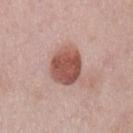Q: Was this lesion biopsied?
A: catalogued during a skin exam; not biopsied
Q: What are the patient's age and sex?
A: male, roughly 55 years of age
Q: What is the anatomic site?
A: the chest
Q: Automated lesion metrics?
A: a lesion area of about 14 mm² and two-axis asymmetry of about 0.2; a lesion color around L≈53 a*≈24 b*≈26 in CIELAB, about 15 CIELAB-L* units darker than the surrounding skin, and a normalized border contrast of about 10
Q: What is the lesion's diameter?
A: ≈4.5 mm
Q: What is the imaging modality?
A: 15 mm crop, total-body photography
Q: Illumination type?
A: white-light